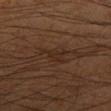notes=catalogued during a skin exam; not biopsied | lesion diameter=about 3.5 mm | anatomic site=the right lower leg | image=~15 mm crop, total-body skin-cancer survey | patient=male, aged 53 to 57.